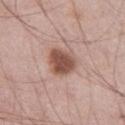diameter: about 3.5 mm
patient: male, approximately 55 years of age
image source: ~15 mm crop, total-body skin-cancer survey
lighting: white-light
site: the leg